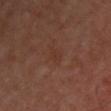biopsy status: total-body-photography surveillance lesion; no biopsy | size: ≈3 mm | subject: male, approximately 50 years of age | location: the front of the torso | TBP lesion metrics: a lesion area of about 3.5 mm², a shape eccentricity near 0.85, and a shape-asymmetry score of about 0.45 (0 = symmetric); an average lesion color of about L≈33 a*≈19 b*≈26 (CIELAB); internal color variation of about 0.5 on a 0–10 scale; lesion-presence confidence of about 100/100 | illumination: cross-polarized | imaging modality: ~15 mm tile from a whole-body skin photo.